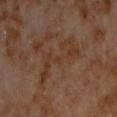Impression: Recorded during total-body skin imaging; not selected for excision or biopsy. Context: A male patient, roughly 60 years of age. Imaged with cross-polarized lighting. From the chest. A 15 mm close-up tile from a total-body photography series done for melanoma screening. The lesion's longest dimension is about 6 mm. An algorithmic analysis of the crop reported a lesion color around L≈32 a*≈17 b*≈26 in CIELAB and a lesion-to-skin contrast of about 5.5 (normalized; higher = more distinct).